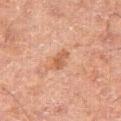Assessment: The lesion was photographed on a routine skin check and not biopsied; there is no pathology result. Clinical summary: The recorded lesion diameter is about 3 mm. Cropped from a total-body skin-imaging series; the visible field is about 15 mm. The lesion-visualizer software estimated an area of roughly 3.5 mm², an eccentricity of roughly 0.85, and a shape-asymmetry score of about 0.3 (0 = symmetric). It also reported an average lesion color of about L≈50 a*≈22 b*≈31 (CIELAB) and a normalized border contrast of about 6.5. It also reported a within-lesion color-variation index near 1.5/10. The software also gave a classifier nevus-likeness of about 5/100 and a lesion-detection confidence of about 100/100. From the leg. A male patient roughly 65 years of age. This is a cross-polarized tile.